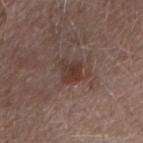The lesion was photographed on a routine skin check and not biopsied; there is no pathology result. A 15 mm close-up tile from a total-body photography series done for melanoma screening. Captured under white-light illumination. The lesion is located on the arm. A male patient aged around 55.